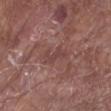Assessment:
No biopsy was performed on this lesion — it was imaged during a full skin examination and was not determined to be concerning.
Background:
This is a white-light tile. A male subject in their mid-60s. A 15 mm close-up tile from a total-body photography series done for melanoma screening. Measured at roughly 3.5 mm in maximum diameter. The total-body-photography lesion software estimated a footprint of about 5.5 mm², an eccentricity of roughly 0.85, and a shape-asymmetry score of about 0.4 (0 = symmetric). It also reported a mean CIELAB color near L≈43 a*≈21 b*≈21 and a normalized border contrast of about 5. And it measured a color-variation rating of about 1/10. The lesion is on the left lower leg.